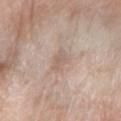Notes:
* follow-up — catalogued during a skin exam; not biopsied
* TBP lesion metrics — an outline eccentricity of about 0.75 (0 = round, 1 = elongated) and a symmetry-axis asymmetry near 0.3; an automated nevus-likeness rating near 0 out of 100 and a detector confidence of about 95 out of 100 that the crop contains a lesion
* anatomic site — the arm
* image source — ~15 mm crop, total-body skin-cancer survey
* illumination — white-light illumination
* subject — female, approximately 70 years of age
* lesion diameter — ≈3 mm